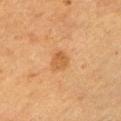Q: Was a biopsy performed?
A: imaged on a skin check; not biopsied
Q: Patient demographics?
A: male, in their 50s
Q: What is the lesion's diameter?
A: about 2.5 mm
Q: Illumination type?
A: cross-polarized
Q: Lesion location?
A: the left upper arm
Q: What kind of image is this?
A: ~15 mm crop, total-body skin-cancer survey